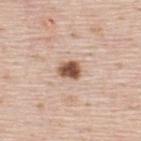Case summary:
– workup — imaged on a skin check; not biopsied
– location — the upper back
– size — about 2.5 mm
– illumination — white-light
– image source — ~15 mm tile from a whole-body skin photo
– patient — male, approximately 45 years of age